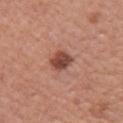{"biopsy_status": "not biopsied; imaged during a skin examination", "patient": {"sex": "female", "age_approx": 60}, "lighting": "white-light", "image": {"source": "total-body photography crop", "field_of_view_mm": 15}, "site": "right upper arm", "lesion_size": {"long_diameter_mm_approx": 3.5}, "automated_metrics": {"area_mm2_approx": 6.0, "eccentricity": 0.6, "vs_skin_darker_L": 14.0, "vs_skin_contrast_norm": 9.5}}